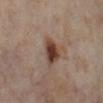A 15 mm crop from a total-body photograph taken for skin-cancer surveillance.
The total-body-photography lesion software estimated a footprint of about 6.5 mm², a shape eccentricity near 0.5, and a shape-asymmetry score of about 0.25 (0 = symmetric). The software also gave an average lesion color of about L≈41 a*≈19 b*≈26 (CIELAB), about 14 CIELAB-L* units darker than the surrounding skin, and a lesion-to-skin contrast of about 11 (normalized; higher = more distinct). And it measured a border-irregularity index near 2.5/10 and radial color variation of about 1.5. And it measured an automated nevus-likeness rating near 100 out of 100 and a lesion-detection confidence of about 100/100.
The patient is a female aged 53 to 57.
The tile uses cross-polarized illumination.
The recorded lesion diameter is about 3.5 mm.
The lesion is located on the left thigh.